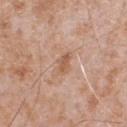Findings:
• workup: no biopsy performed (imaged during a skin exam)